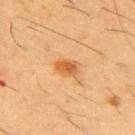No biopsy was performed on this lesion — it was imaged during a full skin examination and was not determined to be concerning. A 15 mm crop from a total-body photograph taken for skin-cancer surveillance. The recorded lesion diameter is about 2.5 mm. A male subject, about 55 years old. Located on the chest. The tile uses cross-polarized illumination.